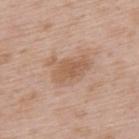Imaged during a routine full-body skin examination; the lesion was not biopsied and no histopathology is available. A male subject aged 48 to 52. A 15 mm close-up tile from a total-body photography series done for melanoma screening. About 5 mm across. Automated image analysis of the tile measured border irregularity of about 4 on a 0–10 scale and a within-lesion color-variation index near 2.5/10. From the upper back.